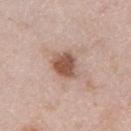<case>
  <biopsy_status>not biopsied; imaged during a skin examination</biopsy_status>
  <lighting>white-light</lighting>
  <site>left upper arm</site>
  <image>
    <source>total-body photography crop</source>
    <field_of_view_mm>15</field_of_view_mm>
  </image>
  <lesion_size>
    <long_diameter_mm_approx>3.0</long_diameter_mm_approx>
  </lesion_size>
  <patient>
    <sex>male</sex>
    <age_approx>40</age_approx>
  </patient>
</case>